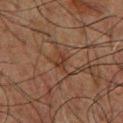Assessment:
Captured during whole-body skin photography for melanoma surveillance; the lesion was not biopsied.
Image and clinical context:
A 15 mm crop from a total-body photograph taken for skin-cancer surveillance. Located on the chest. The patient is a male aged 58–62.